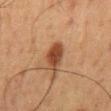Captured during whole-body skin photography for melanoma surveillance; the lesion was not biopsied.
A 15 mm crop from a total-body photograph taken for skin-cancer surveillance.
Measured at roughly 3.5 mm in maximum diameter.
The lesion is on the back.
A male subject aged 58 to 62.
Automated tile analysis of the lesion measured an automated nevus-likeness rating near 100 out of 100 and lesion-presence confidence of about 100/100.